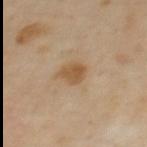Imaged during a routine full-body skin examination; the lesion was not biopsied and no histopathology is available. From the upper back. A close-up tile cropped from a whole-body skin photograph, about 15 mm across. A female subject aged around 60.